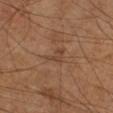{
  "lighting": "cross-polarized",
  "site": "right upper arm",
  "image": {
    "source": "total-body photography crop",
    "field_of_view_mm": 15
  },
  "lesion_size": {
    "long_diameter_mm_approx": 3.0
  },
  "patient": {
    "sex": "male",
    "age_approx": 40
  }
}